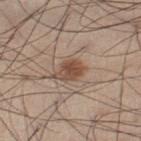Acquisition and patient details: On the left thigh. The total-body-photography lesion software estimated a lesion area of about 7 mm² and a symmetry-axis asymmetry near 0.2. And it measured a mean CIELAB color near L≈49 a*≈18 b*≈27 and a lesion–skin lightness drop of about 11. And it measured a nevus-likeness score of about 90/100 and lesion-presence confidence of about 100/100. This is a white-light tile. The subject is a male in their mid- to late 50s. A lesion tile, about 15 mm wide, cut from a 3D total-body photograph.